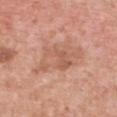Q: Illumination type?
A: white-light illumination
Q: What is the anatomic site?
A: the chest
Q: How was this image acquired?
A: total-body-photography crop, ~15 mm field of view
Q: Lesion size?
A: ≈6.5 mm
Q: What did automated image analysis measure?
A: an area of roughly 16 mm², an eccentricity of roughly 0.85, and a symmetry-axis asymmetry near 0.4; a border-irregularity rating of about 5/10 and a peripheral color-asymmetry measure near 1.5
Q: Who is the patient?
A: female, about 50 years old